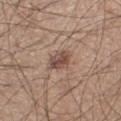Captured during whole-body skin photography for melanoma surveillance; the lesion was not biopsied. A region of skin cropped from a whole-body photographic capture, roughly 15 mm wide. On the left thigh. The total-body-photography lesion software estimated a footprint of about 5 mm² and an eccentricity of roughly 0.6. It also reported a classifier nevus-likeness of about 65/100 and a lesion-detection confidence of about 100/100. The patient is a male in their mid- to late 40s. The tile uses white-light illumination. Longest diameter approximately 2.5 mm.